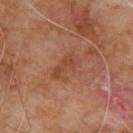Recorded during total-body skin imaging; not selected for excision or biopsy. A lesion tile, about 15 mm wide, cut from a 3D total-body photograph. Located on the upper back. Imaged with cross-polarized lighting. The recorded lesion diameter is about 3.5 mm. A male patient, approximately 65 years of age.